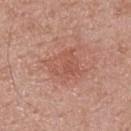workup: catalogued during a skin exam; not biopsied | image source: total-body-photography crop, ~15 mm field of view | patient: male, aged 38–42 | body site: the upper back.